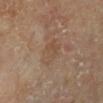biopsy status: total-body-photography surveillance lesion; no biopsy
subject: male, aged 63–67
automated metrics: a lesion area of about 7 mm², a shape eccentricity near 0.75, and two-axis asymmetry of about 0.25; a mean CIELAB color near L≈49 a*≈16 b*≈29 and roughly 6 lightness units darker than nearby skin; an automated nevus-likeness rating near 0 out of 100
image source: ~15 mm crop, total-body skin-cancer survey
location: the left lower leg
tile lighting: cross-polarized